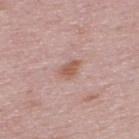The lesion was photographed on a routine skin check and not biopsied; there is no pathology result.
On the back.
A region of skin cropped from a whole-body photographic capture, roughly 15 mm wide.
A male patient, roughly 50 years of age.
The lesion-visualizer software estimated border irregularity of about 2.5 on a 0–10 scale, a within-lesion color-variation index near 2/10, and a peripheral color-asymmetry measure near 0.5. It also reported an automated nevus-likeness rating near 40 out of 100 and lesion-presence confidence of about 100/100.